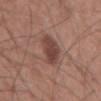The lesion was tiled from a total-body skin photograph and was not biopsied.